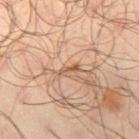workup: no biopsy performed (imaged during a skin exam) | patient: male, roughly 65 years of age | site: the right thigh | image-analysis metrics: a mean CIELAB color near L≈54 a*≈19 b*≈32 and a normalized border contrast of about 7; lesion-presence confidence of about 80/100 | imaging modality: ~15 mm tile from a whole-body skin photo.